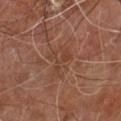Captured during whole-body skin photography for melanoma surveillance; the lesion was not biopsied. Automated image analysis of the tile measured an area of roughly 6 mm² and an eccentricity of roughly 0.85. It also reported a mean CIELAB color near L≈39 a*≈20 b*≈28 and about 5 CIELAB-L* units darker than the surrounding skin. The analysis additionally found a border-irregularity index near 7.5/10, a within-lesion color-variation index near 4/10, and a peripheral color-asymmetry measure near 1.5. And it measured an automated nevus-likeness rating near 0 out of 100 and lesion-presence confidence of about 65/100. This image is a 15 mm lesion crop taken from a total-body photograph. A male patient in their 60s. From the right leg. The recorded lesion diameter is about 4 mm. Imaged with cross-polarized lighting.